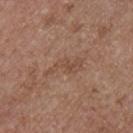No biopsy was performed on this lesion — it was imaged during a full skin examination and was not determined to be concerning. The lesion is located on the arm. Captured under white-light illumination. A 15 mm close-up extracted from a 3D total-body photography capture. The lesion's longest dimension is about 4.5 mm. A male patient aged approximately 55. Automated tile analysis of the lesion measured an area of roughly 6 mm², an outline eccentricity of about 0.9 (0 = round, 1 = elongated), and two-axis asymmetry of about 0.5. The analysis additionally found a mean CIELAB color near L≈48 a*≈19 b*≈28, about 6 CIELAB-L* units darker than the surrounding skin, and a normalized border contrast of about 5. The software also gave a detector confidence of about 100 out of 100 that the crop contains a lesion.